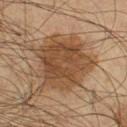notes: no biopsy performed (imaged during a skin exam) | illumination: cross-polarized | size: about 7 mm | location: the leg | patient: male, aged 53–57 | image: total-body-photography crop, ~15 mm field of view | image-analysis metrics: a lesion area of about 28 mm², an outline eccentricity of about 0.45 (0 = round, 1 = elongated), and a symmetry-axis asymmetry near 0.3.